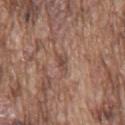Notes:
* notes — catalogued during a skin exam; not biopsied
* subject — male, aged 73 to 77
* TBP lesion metrics — a border-irregularity rating of about 3.5/10, a color-variation rating of about 0/10, and peripheral color asymmetry of about 0; a nevus-likeness score of about 0/100 and a lesion-detection confidence of about 50/100
* body site — the mid back
* image source — ~15 mm crop, total-body skin-cancer survey
* tile lighting — white-light illumination